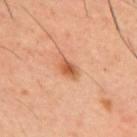Clinical impression: Part of a total-body skin-imaging series; this lesion was reviewed on a skin check and was not flagged for biopsy. Acquisition and patient details: The patient is a male aged approximately 40. A 15 mm close-up tile from a total-body photography series done for melanoma screening. The tile uses cross-polarized illumination. Located on the upper back.